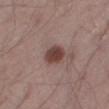Clinical impression:
This lesion was catalogued during total-body skin photography and was not selected for biopsy.
Image and clinical context:
Measured at roughly 3 mm in maximum diameter. A male subject about 55 years old. The lesion-visualizer software estimated a mean CIELAB color near L≈42 a*≈19 b*≈21, a lesion–skin lightness drop of about 13, and a normalized lesion–skin contrast near 10. From the right thigh. This is a white-light tile. Cropped from a whole-body photographic skin survey; the tile spans about 15 mm.